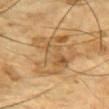Q: Is there a histopathology result?
A: no biopsy performed (imaged during a skin exam)
Q: What is the imaging modality?
A: total-body-photography crop, ~15 mm field of view
Q: Who is the patient?
A: male, roughly 85 years of age or older
Q: What lighting was used for the tile?
A: cross-polarized illumination
Q: Where on the body is the lesion?
A: the chest